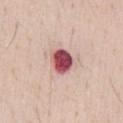Case summary:
- workup — total-body-photography surveillance lesion; no biopsy
- acquisition — ~15 mm tile from a whole-body skin photo
- lesion diameter — ~3 mm (longest diameter)
- anatomic site — the chest
- patient — male, aged around 30
- automated metrics — a lesion area of about 7.5 mm², a shape eccentricity near 0.5, and a shape-asymmetry score of about 0.15 (0 = symmetric); a lesion color around L≈51 a*≈31 b*≈20 in CIELAB, about 22 CIELAB-L* units darker than the surrounding skin, and a lesion-to-skin contrast of about 14 (normalized; higher = more distinct); a nevus-likeness score of about 0/100 and a lesion-detection confidence of about 100/100
- tile lighting — white-light illumination